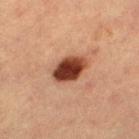No biopsy was performed on this lesion — it was imaged during a full skin examination and was not determined to be concerning.
A female patient, approximately 55 years of age.
On the left thigh.
The lesion's longest dimension is about 4.5 mm.
This image is a 15 mm lesion crop taken from a total-body photograph.
This is a cross-polarized tile.
The total-body-photography lesion software estimated a mean CIELAB color near L≈34 a*≈21 b*≈26 and about 16 CIELAB-L* units darker than the surrounding skin. The analysis additionally found border irregularity of about 1.5 on a 0–10 scale and internal color variation of about 6.5 on a 0–10 scale. It also reported a classifier nevus-likeness of about 100/100 and lesion-presence confidence of about 100/100.